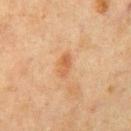{
  "biopsy_status": "not biopsied; imaged during a skin examination"
}